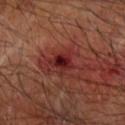This lesion was catalogued during total-body skin photography and was not selected for biopsy. A 15 mm close-up extracted from a 3D total-body photography capture. The subject is a male approximately 65 years of age. Imaged with cross-polarized lighting. From the arm. Automated tile analysis of the lesion measured border irregularity of about 3.5 on a 0–10 scale, a color-variation rating of about 4.5/10, and a peripheral color-asymmetry measure near 1.5.